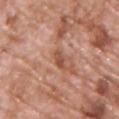workup — imaged on a skin check; not biopsied
subject — male, roughly 70 years of age
anatomic site — the front of the torso
diameter — about 2.5 mm
image source — 15 mm crop, total-body photography